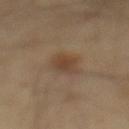Assessment: Part of a total-body skin-imaging series; this lesion was reviewed on a skin check and was not flagged for biopsy. Context: Measured at roughly 3.5 mm in maximum diameter. Automated image analysis of the tile measured a lesion area of about 6.5 mm² and a shape eccentricity near 0.8. It also reported a mean CIELAB color near L≈42 a*≈15 b*≈29, roughly 8 lightness units darker than nearby skin, and a normalized border contrast of about 7. It also reported a border-irregularity rating of about 2.5/10, internal color variation of about 3.5 on a 0–10 scale, and radial color variation of about 1. From the mid back. The patient is a male aged 38–42. A 15 mm crop from a total-body photograph taken for skin-cancer surveillance. Captured under cross-polarized illumination.